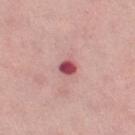{
  "biopsy_status": "not biopsied; imaged during a skin examination",
  "site": "leg",
  "patient": {
    "sex": "female",
    "age_approx": 65
  },
  "image": {
    "source": "total-body photography crop",
    "field_of_view_mm": 15
  }
}